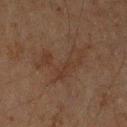Located on the arm.
The tile uses cross-polarized illumination.
Automated image analysis of the tile measured a classifier nevus-likeness of about 0/100 and a lesion-detection confidence of about 100/100.
About 7.5 mm across.
A male patient aged 58 to 62.
This image is a 15 mm lesion crop taken from a total-body photograph.